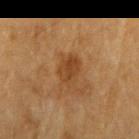notes: catalogued during a skin exam; not biopsied | lesion size: about 3 mm | image-analysis metrics: an area of roughly 6 mm² and a shape-asymmetry score of about 0.2 (0 = symmetric); border irregularity of about 2 on a 0–10 scale and radial color variation of about 1; an automated nevus-likeness rating near 35 out of 100 and a lesion-detection confidence of about 100/100 | tile lighting: cross-polarized | patient: male, aged approximately 85 or older | image: ~15 mm crop, total-body skin-cancer survey | site: the arm.